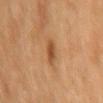Acquisition and patient details:
A roughly 15 mm field-of-view crop from a total-body skin photograph. A female subject aged approximately 55. On the right upper arm. This is a cross-polarized tile.